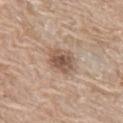No biopsy was performed on this lesion — it was imaged during a full skin examination and was not determined to be concerning. Cropped from a whole-body photographic skin survey; the tile spans about 15 mm. The subject is a female aged around 60. The lesion is on the left thigh.